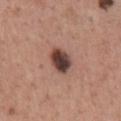The lesion was photographed on a routine skin check and not biopsied; there is no pathology result.
The lesion is on the chest.
Cropped from a total-body skin-imaging series; the visible field is about 15 mm.
An algorithmic analysis of the crop reported an average lesion color of about L≈42 a*≈20 b*≈22 (CIELAB) and a normalized border contrast of about 13. It also reported a border-irregularity rating of about 1.5/10, internal color variation of about 6 on a 0–10 scale, and peripheral color asymmetry of about 1.5. And it measured a lesion-detection confidence of about 100/100.
About 3.5 mm across.
This is a white-light tile.
A male subject in their mid-40s.